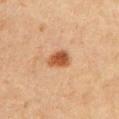Q: Was a biopsy performed?
A: total-body-photography surveillance lesion; no biopsy
Q: Lesion size?
A: about 3 mm
Q: Patient demographics?
A: male, roughly 50 years of age
Q: How was the tile lit?
A: cross-polarized illumination
Q: Where on the body is the lesion?
A: the left upper arm
Q: What did automated image analysis measure?
A: a lesion area of about 5.5 mm², an eccentricity of roughly 0.7, and two-axis asymmetry of about 0.25; border irregularity of about 2 on a 0–10 scale, a within-lesion color-variation index near 3/10, and a peripheral color-asymmetry measure near 1
Q: How was this image acquired?
A: 15 mm crop, total-body photography